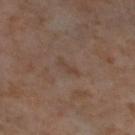Notes:
– workup — no biopsy performed (imaged during a skin exam)
– lighting — cross-polarized
– site — the left thigh
– patient — female, aged 53 to 57
– imaging modality — ~15 mm tile from a whole-body skin photo
– automated lesion analysis — a footprint of about 2 mm², an eccentricity of roughly 0.95, and two-axis asymmetry of about 0.35; an average lesion color of about L≈42 a*≈15 b*≈24 (CIELAB), a lesion–skin lightness drop of about 5, and a normalized lesion–skin contrast near 5; a nevus-likeness score of about 0/100
– size — ≈2.5 mm